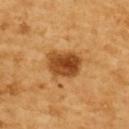  biopsy_status: not biopsied; imaged during a skin examination
  lighting: cross-polarized
  image:
    source: total-body photography crop
    field_of_view_mm: 15
  lesion_size:
    long_diameter_mm_approx: 4.5
  patient:
    sex: female
    age_approx: 55
  site: upper back
  automated_metrics:
    lesion_detection_confidence_0_100: 100